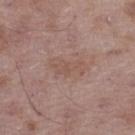Part of a total-body skin-imaging series; this lesion was reviewed on a skin check and was not flagged for biopsy.
A male patient approximately 50 years of age.
Cropped from a total-body skin-imaging series; the visible field is about 15 mm.
From the left thigh.